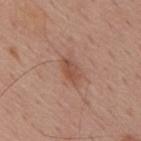Impression: Recorded during total-body skin imaging; not selected for excision or biopsy. Image and clinical context: From the upper back. A male patient aged 58–62. A region of skin cropped from a whole-body photographic capture, roughly 15 mm wide.